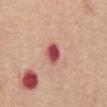The lesion was photographed on a routine skin check and not biopsied; there is no pathology result. The tile uses white-light illumination. An algorithmic analysis of the crop reported an average lesion color of about L≈51 a*≈34 b*≈25 (CIELAB), roughly 18 lightness units darker than nearby skin, and a normalized lesion–skin contrast near 12. And it measured an automated nevus-likeness rating near 0 out of 100. A male subject, aged 63–67. About 3 mm across. This image is a 15 mm lesion crop taken from a total-body photograph. From the abdomen.